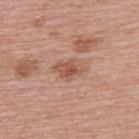  biopsy_status: not biopsied; imaged during a skin examination
  patient:
    sex: female
    age_approx: 60
  lighting: white-light
  image:
    source: total-body photography crop
    field_of_view_mm: 15
  site: upper back
  automated_metrics:
    color_variation_0_10: 4.0
    peripheral_color_asymmetry: 1.0
    nevus_likeness_0_100: 0
    lesion_detection_confidence_0_100: 100